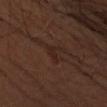biopsy status: total-body-photography surveillance lesion; no biopsy